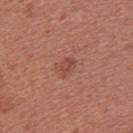Acquisition and patient details: On the upper back. Captured under white-light illumination. Automated image analysis of the tile measured a lesion color around L≈47 a*≈25 b*≈28 in CIELAB and about 7 CIELAB-L* units darker than the surrounding skin. And it measured a nevus-likeness score of about 15/100 and a detector confidence of about 100 out of 100 that the crop contains a lesion. Approximately 2.5 mm at its widest. Cropped from a whole-body photographic skin survey; the tile spans about 15 mm. A female subject aged 23 to 27.